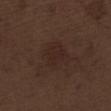Captured during whole-body skin photography for melanoma surveillance; the lesion was not biopsied.
Measured at roughly 3.5 mm in maximum diameter.
A male subject, aged approximately 70.
A region of skin cropped from a whole-body photographic capture, roughly 15 mm wide.
The total-body-photography lesion software estimated a lesion area of about 9.5 mm², a shape eccentricity near 0.45, and two-axis asymmetry of about 0.25. The analysis additionally found border irregularity of about 2.5 on a 0–10 scale.
From the left thigh.